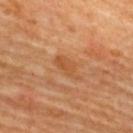Recorded during total-body skin imaging; not selected for excision or biopsy. The lesion is on the upper back. A close-up tile cropped from a whole-body skin photograph, about 15 mm across. A female patient aged 63–67. Captured under cross-polarized illumination.